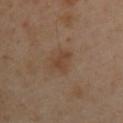The lesion was photographed on a routine skin check and not biopsied; there is no pathology result. From the left upper arm. The lesion-visualizer software estimated an eccentricity of roughly 0.8 and a symmetry-axis asymmetry near 0.4. The software also gave a lesion color around L≈42 a*≈17 b*≈29 in CIELAB and a lesion–skin lightness drop of about 7. The analysis additionally found a detector confidence of about 100 out of 100 that the crop contains a lesion. A roughly 15 mm field-of-view crop from a total-body skin photograph. The subject is a female aged 38–42.